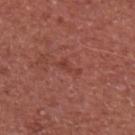Clinical impression:
The lesion was tiled from a total-body skin photograph and was not biopsied.
Background:
A 15 mm close-up extracted from a 3D total-body photography capture. A male patient aged 53–57. The lesion is located on the right upper arm.